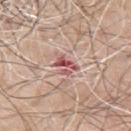Impression:
Imaged during a routine full-body skin examination; the lesion was not biopsied and no histopathology is available.
Clinical summary:
The lesion is located on the chest. Automated tile analysis of the lesion measured an area of roughly 4.5 mm² and an outline eccentricity of about 0.75 (0 = round, 1 = elongated). Measured at roughly 3 mm in maximum diameter. The tile uses white-light illumination. A male subject approximately 70 years of age. A 15 mm crop from a total-body photograph taken for skin-cancer surveillance.